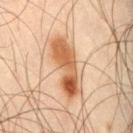Q: Was a biopsy performed?
A: total-body-photography surveillance lesion; no biopsy
Q: Where on the body is the lesion?
A: the right thigh
Q: Who is the patient?
A: male, roughly 45 years of age
Q: How was this image acquired?
A: ~15 mm tile from a whole-body skin photo